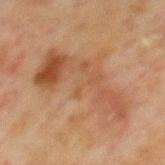Recorded during total-body skin imaging; not selected for excision or biopsy.
A 15 mm crop from a total-body photograph taken for skin-cancer surveillance.
Longest diameter approximately 10.5 mm.
Located on the mid back.
A male subject approximately 70 years of age.
The tile uses cross-polarized illumination.